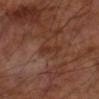Impression: Part of a total-body skin-imaging series; this lesion was reviewed on a skin check and was not flagged for biopsy. Clinical summary: Automated tile analysis of the lesion measured a lesion color around L≈32 a*≈22 b*≈28 in CIELAB, a lesion–skin lightness drop of about 6, and a normalized lesion–skin contrast near 6.5. The software also gave a border-irregularity rating of about 5.5/10, a within-lesion color-variation index near 0/10, and peripheral color asymmetry of about 0. Imaged with cross-polarized lighting. About 3.5 mm across. A lesion tile, about 15 mm wide, cut from a 3D total-body photograph. The lesion is on the left upper arm. A male subject approximately 70 years of age.